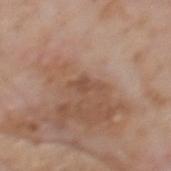This lesion was catalogued during total-body skin photography and was not selected for biopsy. Automated tile analysis of the lesion measured an average lesion color of about L≈49 a*≈20 b*≈29 (CIELAB), roughly 8 lightness units darker than nearby skin, and a normalized lesion–skin contrast near 6. The analysis additionally found a border-irregularity index near 5/10, internal color variation of about 0.5 on a 0–10 scale, and radial color variation of about 0. And it measured a classifier nevus-likeness of about 0/100 and lesion-presence confidence of about 100/100. Longest diameter approximately 3.5 mm. The subject is a male approximately 75 years of age. A close-up tile cropped from a whole-body skin photograph, about 15 mm across. Located on the back. The tile uses white-light illumination.